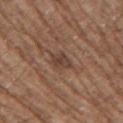Captured during whole-body skin photography for melanoma surveillance; the lesion was not biopsied. About 3 mm across. The subject is a male approximately 70 years of age. A 15 mm close-up tile from a total-body photography series done for melanoma screening. The lesion is located on the right upper arm. Automated image analysis of the tile measured a mean CIELAB color near L≈41 a*≈18 b*≈25, a lesion–skin lightness drop of about 9, and a normalized lesion–skin contrast near 7. The analysis additionally found an automated nevus-likeness rating near 0 out of 100 and a detector confidence of about 85 out of 100 that the crop contains a lesion.